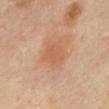Clinical impression:
No biopsy was performed on this lesion — it was imaged during a full skin examination and was not determined to be concerning.
Background:
On the mid back. Captured under cross-polarized illumination. The patient is a female in their 60s. About 3.5 mm across. An algorithmic analysis of the crop reported a lesion–skin lightness drop of about 6 and a lesion-to-skin contrast of about 5 (normalized; higher = more distinct). The software also gave a border-irregularity rating of about 2.5/10, a color-variation rating of about 2/10, and peripheral color asymmetry of about 0.5. It also reported an automated nevus-likeness rating near 100 out of 100 and lesion-presence confidence of about 100/100. This image is a 15 mm lesion crop taken from a total-body photograph.